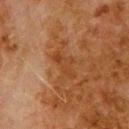The lesion was tiled from a total-body skin photograph and was not biopsied.
A male patient, roughly 80 years of age.
Captured under cross-polarized illumination.
Cropped from a whole-body photographic skin survey; the tile spans about 15 mm.
On the upper back.